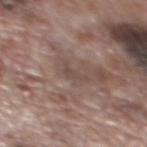| key | value |
|---|---|
| anatomic site | the mid back |
| acquisition | 15 mm crop, total-body photography |
| image-analysis metrics | a footprint of about 2.5 mm², a shape eccentricity near 0.85, and a shape-asymmetry score of about 0.55 (0 = symmetric); a mean CIELAB color near L≈46 a*≈15 b*≈21 and a lesion-to-skin contrast of about 5 (normalized; higher = more distinct); an automated nevus-likeness rating near 0 out of 100 |
| subject | male, in their 70s |
| lesion size | ≈2.5 mm |
| lighting | white-light illumination |